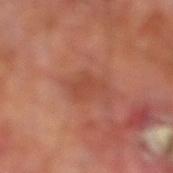Q: Is there a histopathology result?
A: no biopsy performed (imaged during a skin exam)
Q: What are the patient's age and sex?
A: male, aged approximately 70
Q: What is the lesion's diameter?
A: ≈4 mm
Q: What is the imaging modality?
A: total-body-photography crop, ~15 mm field of view
Q: What is the anatomic site?
A: the right lower leg
Q: What lighting was used for the tile?
A: cross-polarized illumination